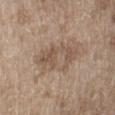Q: Lesion location?
A: the leg
Q: What is the lesion's diameter?
A: ~6 mm (longest diameter)
Q: How was this image acquired?
A: ~15 mm tile from a whole-body skin photo
Q: What are the patient's age and sex?
A: male, about 70 years old
Q: Illumination type?
A: white-light